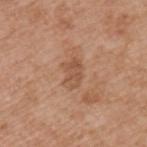follow-up: total-body-photography surveillance lesion; no biopsy
location: the upper back
image: ~15 mm tile from a whole-body skin photo
patient: male, aged 53–57